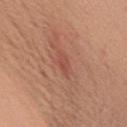biopsy_status: not biopsied; imaged during a skin examination
patient:
  sex: female
  age_approx: 55
image:
  source: total-body photography crop
  field_of_view_mm: 15
lighting: white-light
site: upper back
lesion_size:
  long_diameter_mm_approx: 3.0